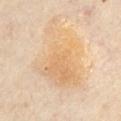Notes:
- biopsy status · no biopsy performed (imaged during a skin exam)
- site · the chest
- subject · female, in their 60s
- image · 15 mm crop, total-body photography